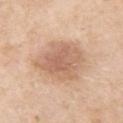Context: The lesion's longest dimension is about 6 mm. A female subject in their mid-70s. The total-body-photography lesion software estimated an area of roughly 22 mm² and a shape eccentricity near 0.5. It also reported border irregularity of about 2 on a 0–10 scale and radial color variation of about 1. And it measured a nevus-likeness score of about 25/100. A close-up tile cropped from a whole-body skin photograph, about 15 mm across. The tile uses white-light illumination. Located on the arm.